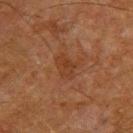The recorded lesion diameter is about 3 mm. The tile uses cross-polarized illumination. Automated tile analysis of the lesion measured an area of roughly 4.5 mm², an outline eccentricity of about 0.7 (0 = round, 1 = elongated), and a shape-asymmetry score of about 0.3 (0 = symmetric). The software also gave a border-irregularity index near 3.5/10 and internal color variation of about 2 on a 0–10 scale. The analysis additionally found a lesion-detection confidence of about 100/100. Cropped from a whole-body photographic skin survey; the tile spans about 15 mm. From the upper back. A male subject about 65 years old.